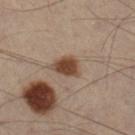Q: What kind of image is this?
A: ~15 mm tile from a whole-body skin photo
Q: What are the patient's age and sex?
A: male, aged approximately 55
Q: Where on the body is the lesion?
A: the leg
Q: How was the tile lit?
A: cross-polarized illumination
Q: What did automated image analysis measure?
A: a footprint of about 6.5 mm², a shape eccentricity near 0.7, and two-axis asymmetry of about 0.3; a lesion color around L≈35 a*≈14 b*≈23 in CIELAB, roughly 11 lightness units darker than nearby skin, and a lesion-to-skin contrast of about 10.5 (normalized; higher = more distinct); a classifier nevus-likeness of about 100/100 and lesion-presence confidence of about 100/100